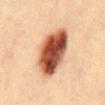| feature | finding |
|---|---|
| histopathology | a dysplastic (Clark) nevus |